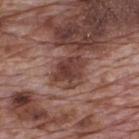Captured during whole-body skin photography for melanoma surveillance; the lesion was not biopsied.
A roughly 15 mm field-of-view crop from a total-body skin photograph.
The lesion is located on the upper back.
A male patient, aged 68 to 72.